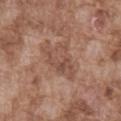The lesion is located on the abdomen. The recorded lesion diameter is about 5.5 mm. Cropped from a whole-body photographic skin survey; the tile spans about 15 mm. The lesion-visualizer software estimated an area of roughly 12 mm², a shape eccentricity near 0.8, and a symmetry-axis asymmetry near 0.4. And it measured an average lesion color of about L≈50 a*≈20 b*≈27 (CIELAB), about 7 CIELAB-L* units darker than the surrounding skin, and a lesion-to-skin contrast of about 5.5 (normalized; higher = more distinct). It also reported a border-irregularity rating of about 5/10 and radial color variation of about 1.5. A male patient aged around 75.